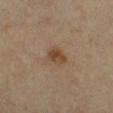biopsy status = no biopsy performed (imaged during a skin exam); site = the right lower leg; subject = male, aged approximately 65; automated metrics = an automated nevus-likeness rating near 70 out of 100; illumination = cross-polarized illumination; lesion size = ~2.5 mm (longest diameter); image source = ~15 mm tile from a whole-body skin photo.